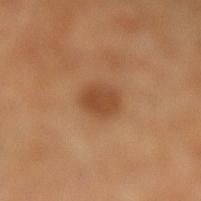The lesion was photographed on a routine skin check and not biopsied; there is no pathology result. From the leg. A male patient aged 58 to 62. Approximately 3 mm at its widest. Captured under cross-polarized illumination. A 15 mm crop from a total-body photograph taken for skin-cancer surveillance. Automated tile analysis of the lesion measured a footprint of about 6 mm², an outline eccentricity of about 0.45 (0 = round, 1 = elongated), and two-axis asymmetry of about 0.1. It also reported internal color variation of about 2 on a 0–10 scale and peripheral color asymmetry of about 1. The software also gave a classifier nevus-likeness of about 70/100 and a detector confidence of about 100 out of 100 that the crop contains a lesion.